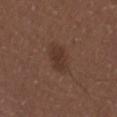Acquisition and patient details:
A lesion tile, about 15 mm wide, cut from a 3D total-body photograph. The tile uses white-light illumination. A male patient aged approximately 30. From the right upper arm.